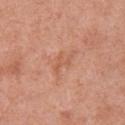Captured during whole-body skin photography for melanoma surveillance; the lesion was not biopsied. The lesion is located on the chest. A lesion tile, about 15 mm wide, cut from a 3D total-body photograph. Captured under white-light illumination. A male subject aged 68–72.